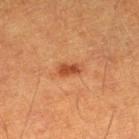This lesion was catalogued during total-body skin photography and was not selected for biopsy.
A 15 mm close-up tile from a total-body photography series done for melanoma screening.
A male subject, aged around 60.
The lesion is on the left thigh.